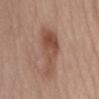workup = catalogued during a skin exam; not biopsied
lighting = white-light
patient = female, aged approximately 50
diameter = ≈8 mm
location = the abdomen
imaging modality = ~15 mm tile from a whole-body skin photo